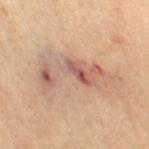| feature | finding |
|---|---|
| notes | catalogued during a skin exam; not biopsied |
| tile lighting | cross-polarized illumination |
| imaging modality | ~15 mm tile from a whole-body skin photo |
| subject | female, approximately 65 years of age |
| lesion diameter | ~8.5 mm (longest diameter) |
| anatomic site | the left leg |